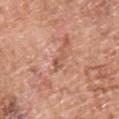No biopsy was performed on this lesion — it was imaged during a full skin examination and was not determined to be concerning. A 15 mm close-up tile from a total-body photography series done for melanoma screening. Imaged with white-light lighting. On the upper back. The subject is a male aged 53–57. About 3 mm across.